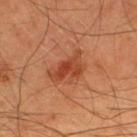Acquisition and patient details: The lesion is located on the back. A male subject, aged 43–47. A 15 mm close-up extracted from a 3D total-body photography capture.